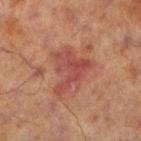  biopsy_status: not biopsied; imaged during a skin examination